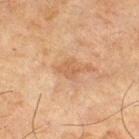tile lighting: cross-polarized | image source: ~15 mm tile from a whole-body skin photo | size: ~2.5 mm (longest diameter) | subject: male, in their mid-60s | body site: the right thigh | TBP lesion metrics: a lesion color around L≈47 a*≈18 b*≈30 in CIELAB, about 6 CIELAB-L* units darker than the surrounding skin, and a normalized border contrast of about 5.5.